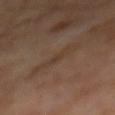• biopsy status — no biopsy performed (imaged during a skin exam)
• TBP lesion metrics — two-axis asymmetry of about 0.3; an average lesion color of about L≈36 a*≈15 b*≈25 (CIELAB), about 4 CIELAB-L* units darker than the surrounding skin, and a normalized border contrast of about 4.5; a border-irregularity index near 5/10 and internal color variation of about 2.5 on a 0–10 scale
• image source — ~15 mm crop, total-body skin-cancer survey
• illumination — cross-polarized illumination
• location — the mid back
• subject — male, aged 68 to 72